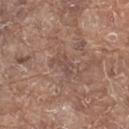The lesion was tiled from a total-body skin photograph and was not biopsied.
On the upper back.
Measured at roughly 3 mm in maximum diameter.
A male patient about 80 years old.
A region of skin cropped from a whole-body photographic capture, roughly 15 mm wide.